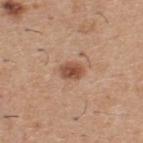No biopsy was performed on this lesion — it was imaged during a full skin examination and was not determined to be concerning. Approximately 2.5 mm at its widest. The lesion is located on the back. A 15 mm close-up extracted from a 3D total-body photography capture. The patient is a male aged 58–62.